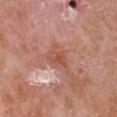notes: total-body-photography surveillance lesion; no biopsy | anatomic site: the right upper arm | tile lighting: white-light illumination | lesion size: about 4 mm | subject: male, roughly 65 years of age | imaging modality: ~15 mm crop, total-body skin-cancer survey.